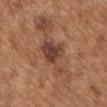Assessment: Imaged during a routine full-body skin examination; the lesion was not biopsied and no histopathology is available. Context: The patient is a male aged approximately 65. On the chest. A 15 mm crop from a total-body photograph taken for skin-cancer surveillance. The lesion-visualizer software estimated an area of roughly 33 mm², a shape eccentricity near 0.9, and two-axis asymmetry of about 0.35. The software also gave an average lesion color of about L≈47 a*≈21 b*≈29 (CIELAB), roughly 8 lightness units darker than nearby skin, and a lesion-to-skin contrast of about 6.5 (normalized; higher = more distinct). The software also gave a border-irregularity rating of about 7/10, a color-variation rating of about 7/10, and a peripheral color-asymmetry measure near 2. Longest diameter approximately 10 mm. This is a white-light tile.